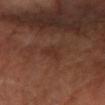The lesion was photographed on a routine skin check and not biopsied; there is no pathology result. The lesion-visualizer software estimated a lesion area of about 3.5 mm² and a symmetry-axis asymmetry near 0.4. It also reported a border-irregularity index near 4/10, internal color variation of about 1 on a 0–10 scale, and peripheral color asymmetry of about 0.5. This is a cross-polarized tile. On the left forearm. A roughly 15 mm field-of-view crop from a total-body skin photograph. A female subject, aged around 70. Measured at roughly 2.5 mm in maximum diameter.